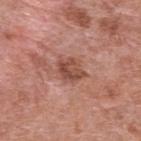No biopsy was performed on this lesion — it was imaged during a full skin examination and was not determined to be concerning. An algorithmic analysis of the crop reported an average lesion color of about L≈49 a*≈24 b*≈28 (CIELAB), about 11 CIELAB-L* units darker than the surrounding skin, and a normalized lesion–skin contrast near 7.5. The analysis additionally found an automated nevus-likeness rating near 20 out of 100 and a lesion-detection confidence of about 100/100. The lesion is on the back. A female patient aged 68 to 72. This image is a 15 mm lesion crop taken from a total-body photograph.